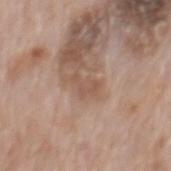Part of a total-body skin-imaging series; this lesion was reviewed on a skin check and was not flagged for biopsy. The patient is a male aged 68 to 72. The lesion is located on the mid back. Captured under white-light illumination. Cropped from a whole-body photographic skin survey; the tile spans about 15 mm. Automated image analysis of the tile measured an outline eccentricity of about 0.85 (0 = round, 1 = elongated) and a shape-asymmetry score of about 0.55 (0 = symmetric). The analysis additionally found a nevus-likeness score of about 0/100 and a lesion-detection confidence of about 100/100.